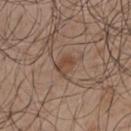Impression: Recorded during total-body skin imaging; not selected for excision or biopsy.